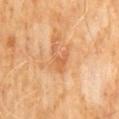| key | value |
|---|---|
| follow-up | no biopsy performed (imaged during a skin exam) |
| image source | ~15 mm tile from a whole-body skin photo |
| subject | male, approximately 60 years of age |
| site | the abdomen |